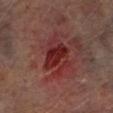Assessment: Captured during whole-body skin photography for melanoma surveillance; the lesion was not biopsied. Background: A 15 mm close-up tile from a total-body photography series done for melanoma screening. Located on the left lower leg. The total-body-photography lesion software estimated a mean CIELAB color near L≈29 a*≈29 b*≈23 and a normalized lesion–skin contrast near 9.5. It also reported border irregularity of about 4 on a 0–10 scale and peripheral color asymmetry of about 1.5. And it measured a classifier nevus-likeness of about 0/100. A male subject, about 65 years old. This is a cross-polarized tile.